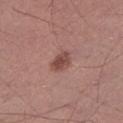Context: Cropped from a whole-body photographic skin survey; the tile spans about 15 mm. Automated image analysis of the tile measured a mean CIELAB color near L≈47 a*≈22 b*≈24. The analysis additionally found a border-irregularity rating of about 2.5/10, internal color variation of about 2.5 on a 0–10 scale, and peripheral color asymmetry of about 1. It also reported an automated nevus-likeness rating near 85 out of 100. Captured under white-light illumination. Located on the left lower leg. The recorded lesion diameter is about 3 mm. A male subject approximately 30 years of age.